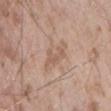| field | value |
|---|---|
| follow-up | catalogued during a skin exam; not biopsied |
| lesion diameter | ~3.5 mm (longest diameter) |
| image | ~15 mm tile from a whole-body skin photo |
| tile lighting | white-light |
| TBP lesion metrics | lesion-presence confidence of about 95/100 |
| subject | male, in their mid- to late 50s |
| location | the lower back |